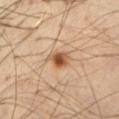Q: Was a biopsy performed?
A: no biopsy performed (imaged during a skin exam)
Q: What is the anatomic site?
A: the front of the torso
Q: How was the tile lit?
A: cross-polarized
Q: Automated lesion metrics?
A: a lesion area of about 5 mm² and a shape-asymmetry score of about 0.35 (0 = symmetric); a border-irregularity rating of about 3/10, internal color variation of about 5.5 on a 0–10 scale, and radial color variation of about 1.5; a nevus-likeness score of about 95/100
Q: What is the imaging modality?
A: total-body-photography crop, ~15 mm field of view
Q: What are the patient's age and sex?
A: male, aged approximately 35